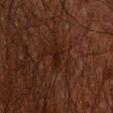{
  "biopsy_status": "not biopsied; imaged during a skin examination",
  "patient": {
    "sex": "male",
    "age_approx": 60
  },
  "image": {
    "source": "total-body photography crop",
    "field_of_view_mm": 15
  },
  "site": "arm"
}